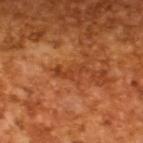Part of a total-body skin-imaging series; this lesion was reviewed on a skin check and was not flagged for biopsy. A roughly 15 mm field-of-view crop from a total-body skin photograph. A male patient, about 65 years old. This is a cross-polarized tile. The recorded lesion diameter is about 4 mm.